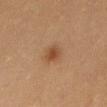{"biopsy_status": "not biopsied; imaged during a skin examination", "patient": {"sex": "female", "age_approx": 30}, "site": "lower back", "lighting": "cross-polarized", "image": {"source": "total-body photography crop", "field_of_view_mm": 15}}